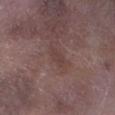Clinical impression: This lesion was catalogued during total-body skin photography and was not selected for biopsy. Clinical summary: A close-up tile cropped from a whole-body skin photograph, about 15 mm across. The lesion is located on the left lower leg. About 3 mm across. A male subject in their mid- to late 70s.